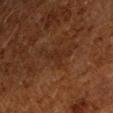Notes:
* notes — no biopsy performed (imaged during a skin exam)
* lighting — cross-polarized
* imaging modality — ~15 mm tile from a whole-body skin photo
* patient — male, aged 58–62
* size — about 3 mm
* location — the left forearm